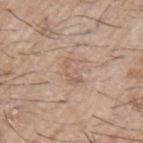<lesion>
<biopsy_status>not biopsied; imaged during a skin examination</biopsy_status>
<automated_metrics>
  <area_mm2_approx>4.5</area_mm2_approx>
  <eccentricity>0.8</eccentricity>
  <shape_asymmetry>0.45</shape_asymmetry>
  <cielab_L>59</cielab_L>
  <cielab_a>17</cielab_a>
  <cielab_b>29</cielab_b>
  <vs_skin_darker_L>7.0</vs_skin_darker_L>
  <vs_skin_contrast_norm>4.5</vs_skin_contrast_norm>
  <nevus_likeness_0_100>0</nevus_likeness_0_100>
  <lesion_detection_confidence_0_100>100</lesion_detection_confidence_0_100>
</automated_metrics>
<lesion_size>
  <long_diameter_mm_approx>3.0</long_diameter_mm_approx>
</lesion_size>
<image>
  <source>total-body photography crop</source>
  <field_of_view_mm>15</field_of_view_mm>
</image>
<patient>
  <sex>male</sex>
  <age_approx>65</age_approx>
</patient>
<site>left upper arm</site>
<lighting>white-light</lighting>
</lesion>